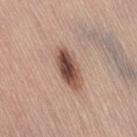Notes:
* follow-up — total-body-photography surveillance lesion; no biopsy
* diameter — ≈5 mm
* imaging modality — ~15 mm crop, total-body skin-cancer survey
* site — the back
* patient — female, aged 63–67
* tile lighting — white-light